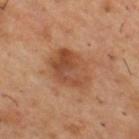  biopsy_status: not biopsied; imaged during a skin examination
  patient:
    sex: male
    age_approx: 55
  site: upper back
  lighting: cross-polarized
  automated_metrics:
    area_mm2_approx: 16.0
    eccentricity: 0.7
    shape_asymmetry: 0.2
    cielab_L: 47
    cielab_a: 22
    cielab_b: 33
    vs_skin_darker_L: 9.0
    vs_skin_contrast_norm: 7.0
    nevus_likeness_0_100: 30
    lesion_detection_confidence_0_100: 100
  image:
    source: total-body photography crop
    field_of_view_mm: 15